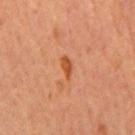| key | value |
|---|---|
| biopsy status | no biopsy performed (imaged during a skin exam) |
| subject | male, aged 63–67 |
| body site | the mid back |
| image | 15 mm crop, total-body photography |